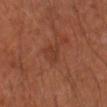• follow-up · imaged on a skin check; not biopsied
• image source · ~15 mm crop, total-body skin-cancer survey
• size · ~2.5 mm (longest diameter)
• patient · male, aged around 65
• tile lighting · cross-polarized illumination
• body site · the right upper arm